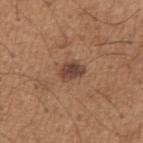The lesion was photographed on a routine skin check and not biopsied; there is no pathology result. A male subject approximately 65 years of age. The tile uses white-light illumination. This image is a 15 mm lesion crop taken from a total-body photograph. From the right upper arm.